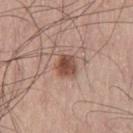The lesion was photographed on a routine skin check and not biopsied; there is no pathology result. A roughly 15 mm field-of-view crop from a total-body skin photograph. From the left thigh. This is a white-light tile. A male subject aged approximately 60. Longest diameter approximately 2.5 mm.